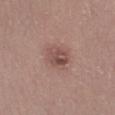Notes:
* notes · imaged on a skin check; not biopsied
* site · the left lower leg
* lighting · white-light
* image-analysis metrics · two-axis asymmetry of about 0.15; a border-irregularity index near 1.5/10, internal color variation of about 5.5 on a 0–10 scale, and peripheral color asymmetry of about 2; a nevus-likeness score of about 35/100 and a lesion-detection confidence of about 100/100
* subject · female, roughly 40 years of age
* lesion size · ≈3.5 mm
* image · ~15 mm crop, total-body skin-cancer survey